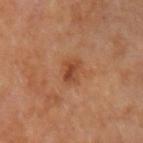Assessment: The lesion was photographed on a routine skin check and not biopsied; there is no pathology result.